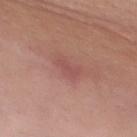{
  "biopsy_status": "not biopsied; imaged during a skin examination",
  "site": "chest",
  "automated_metrics": {
    "area_mm2_approx": 3.0,
    "eccentricity": 0.85,
    "cielab_L": 51,
    "cielab_a": 26,
    "cielab_b": 23,
    "vs_skin_darker_L": 6.0,
    "vs_skin_contrast_norm": 5.0,
    "nevus_likeness_0_100": 0,
    "lesion_detection_confidence_0_100": 100
  },
  "lesion_size": {
    "long_diameter_mm_approx": 2.5
  },
  "lighting": "white-light",
  "patient": {
    "sex": "female",
    "age_approx": 40
  },
  "image": {
    "source": "total-body photography crop",
    "field_of_view_mm": 15
  }
}